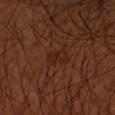Impression: Part of a total-body skin-imaging series; this lesion was reviewed on a skin check and was not flagged for biopsy. Clinical summary: Captured under cross-polarized illumination. Approximately 3 mm at its widest. Cropped from a total-body skin-imaging series; the visible field is about 15 mm. A male patient roughly 50 years of age. On the left arm. An algorithmic analysis of the crop reported an outline eccentricity of about 0.8 (0 = round, 1 = elongated) and two-axis asymmetry of about 0.35. And it measured about 4 CIELAB-L* units darker than the surrounding skin and a normalized border contrast of about 5. The software also gave a border-irregularity index near 3.5/10, a within-lesion color-variation index near 2.5/10, and peripheral color asymmetry of about 1. It also reported a nevus-likeness score of about 5/100 and a detector confidence of about 100 out of 100 that the crop contains a lesion.